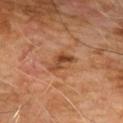Recorded during total-body skin imaging; not selected for excision or biopsy.
The tile uses cross-polarized illumination.
The lesion-visualizer software estimated a lesion area of about 4.5 mm² and an outline eccentricity of about 0.8 (0 = round, 1 = elongated). It also reported a lesion color around L≈43 a*≈23 b*≈34 in CIELAB, roughly 11 lightness units darker than nearby skin, and a normalized lesion–skin contrast near 8. The analysis additionally found a border-irregularity index near 5/10 and a within-lesion color-variation index near 3/10.
On the chest.
The recorded lesion diameter is about 3.5 mm.
A male patient in their 60s.
Cropped from a whole-body photographic skin survey; the tile spans about 15 mm.